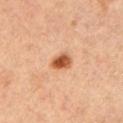biopsy status: total-body-photography surveillance lesion; no biopsy | lighting: cross-polarized illumination | acquisition: ~15 mm crop, total-body skin-cancer survey | site: the left upper arm | patient: male, roughly 55 years of age.